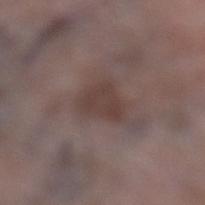Assessment:
This lesion was catalogued during total-body skin photography and was not selected for biopsy.
Background:
Located on the left lower leg. A female patient, aged approximately 70. A region of skin cropped from a whole-body photographic capture, roughly 15 mm wide. The tile uses white-light illumination.